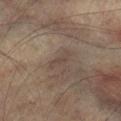Q: Is there a histopathology result?
A: imaged on a skin check; not biopsied
Q: Lesion location?
A: the right lower leg
Q: Patient demographics?
A: male, roughly 75 years of age
Q: What kind of image is this?
A: 15 mm crop, total-body photography
Q: How was the tile lit?
A: cross-polarized illumination
Q: What is the lesion's diameter?
A: ~3 mm (longest diameter)